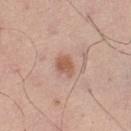Case summary:
- biopsy status: total-body-photography surveillance lesion; no biopsy
- lesion size: ~2.5 mm (longest diameter)
- site: the left thigh
- automated lesion analysis: a lesion area of about 4 mm², a shape eccentricity near 0.65, and a shape-asymmetry score of about 0.3 (0 = symmetric); a color-variation rating of about 1.5/10
- subject: male, in their 70s
- lighting: white-light
- image source: ~15 mm crop, total-body skin-cancer survey